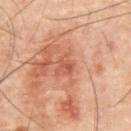Acquisition and patient details:
A region of skin cropped from a whole-body photographic capture, roughly 15 mm wide. Automated image analysis of the tile measured an area of roughly 3.5 mm², an outline eccentricity of about 0.5 (0 = round, 1 = elongated), and two-axis asymmetry of about 0.2. The analysis additionally found a detector confidence of about 100 out of 100 that the crop contains a lesion. The lesion is located on the abdomen. A male subject about 60 years old.